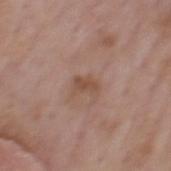follow-up — imaged on a skin check; not biopsied | patient — male, aged 73 to 77 | body site — the mid back | image — 15 mm crop, total-body photography | automated lesion analysis — an area of roughly 4 mm², an eccentricity of roughly 0.75, and two-axis asymmetry of about 0.3; a lesion color around L≈49 a*≈19 b*≈28 in CIELAB, a lesion–skin lightness drop of about 7, and a lesion-to-skin contrast of about 6.5 (normalized; higher = more distinct); a color-variation rating of about 2/10 and radial color variation of about 0.5.